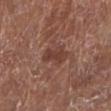Q: Is there a histopathology result?
A: total-body-photography surveillance lesion; no biopsy
Q: Patient demographics?
A: female, about 80 years old
Q: How was the tile lit?
A: white-light
Q: What is the anatomic site?
A: the left lower leg
Q: What kind of image is this?
A: ~15 mm crop, total-body skin-cancer survey
Q: How large is the lesion?
A: ≈3.5 mm
Q: What did automated image analysis measure?
A: an eccentricity of roughly 0.85 and two-axis asymmetry of about 0.35; a detector confidence of about 100 out of 100 that the crop contains a lesion